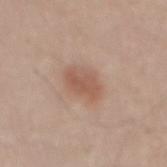workup = no biopsy performed (imaged during a skin exam) | patient = male, in their mid- to late 40s | location = the mid back | image source = ~15 mm crop, total-body skin-cancer survey | illumination = white-light | size = ≈3.5 mm.